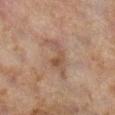Clinical impression:
Captured during whole-body skin photography for melanoma surveillance; the lesion was not biopsied.
Image and clinical context:
Located on the right lower leg. A roughly 15 mm field-of-view crop from a total-body skin photograph. A female subject aged 58 to 62. Captured under cross-polarized illumination. Measured at roughly 5 mm in maximum diameter.